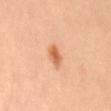workup: no biopsy performed (imaged during a skin exam)
automated metrics: an area of roughly 7 mm², a shape eccentricity near 0.8, and a symmetry-axis asymmetry near 0.2; a mean CIELAB color near L≈66 a*≈23 b*≈38, a lesion–skin lightness drop of about 9, and a normalized border contrast of about 6; a border-irregularity rating of about 2/10 and a peripheral color-asymmetry measure near 1.5; a classifier nevus-likeness of about 95/100
image source: ~15 mm tile from a whole-body skin photo
subject: female, approximately 50 years of age
diameter: ~3.5 mm (longest diameter)
illumination: cross-polarized